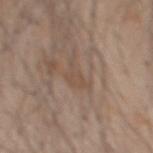This is a white-light tile.
The subject is a male aged 58–62.
The total-body-photography lesion software estimated a lesion area of about 4.5 mm², an outline eccentricity of about 0.85 (0 = round, 1 = elongated), and a symmetry-axis asymmetry near 0.55. The software also gave a border-irregularity rating of about 7/10. The software also gave a classifier nevus-likeness of about 0/100 and lesion-presence confidence of about 65/100.
Located on the mid back.
Approximately 4 mm at its widest.
A close-up tile cropped from a whole-body skin photograph, about 15 mm across.